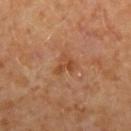| key | value |
|---|---|
| workup | imaged on a skin check; not biopsied |
| tile lighting | cross-polarized |
| anatomic site | the right forearm |
| TBP lesion metrics | a footprint of about 4 mm²; a lesion color around L≈45 a*≈23 b*≈35 in CIELAB, roughly 7 lightness units darker than nearby skin, and a normalized border contrast of about 6.5 |
| subject | female, approximately 50 years of age |
| acquisition | ~15 mm crop, total-body skin-cancer survey |